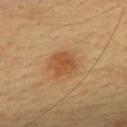  biopsy_status: not biopsied; imaged during a skin examination
  lighting: cross-polarized
  image:
    source: total-body photography crop
    field_of_view_mm: 15
  lesion_size:
    long_diameter_mm_approx: 3.0
  site: upper back
  patient:
    sex: female
    age_approx: 30
  automated_metrics:
    border_irregularity_0_10: 2.0
    color_variation_0_10: 2.5
    peripheral_color_asymmetry: 1.0
    nevus_likeness_0_100: 95